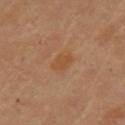biopsy_status: not biopsied; imaged during a skin examination
lighting: cross-polarized
image:
  source: total-body photography crop
  field_of_view_mm: 15
patient:
  sex: female
  age_approx: 60
site: left upper arm
lesion_size:
  long_diameter_mm_approx: 2.5